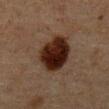Imaged during a routine full-body skin examination; the lesion was not biopsied and no histopathology is available.
The lesion is located on the abdomen.
Captured under cross-polarized illumination.
Approximately 5.5 mm at its widest.
The subject is a male in their mid- to late 70s.
Automated image analysis of the tile measured a border-irregularity rating of about 2/10.
A 15 mm crop from a total-body photograph taken for skin-cancer surveillance.